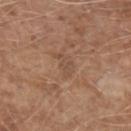Image and clinical context:
The lesion-visualizer software estimated a footprint of about 3.5 mm², an eccentricity of roughly 0.85, and a shape-asymmetry score of about 0.45 (0 = symmetric). It also reported a lesion color around L≈49 a*≈18 b*≈28 in CIELAB and a normalized lesion–skin contrast near 4.5. The software also gave lesion-presence confidence of about 95/100. Captured under white-light illumination. The recorded lesion diameter is about 3 mm. The lesion is located on the right upper arm. A male patient aged 58 to 62. This image is a 15 mm lesion crop taken from a total-body photograph.